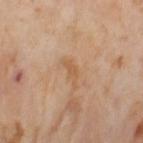No biopsy was performed on this lesion — it was imaged during a full skin examination and was not determined to be concerning. This is a cross-polarized tile. A 15 mm close-up tile from a total-body photography series done for melanoma screening. The lesion is on the left thigh. Approximately 3 mm at its widest. The subject is a female approximately 55 years of age.